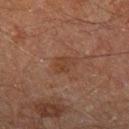Automated image analysis of the tile measured a border-irregularity index near 3.5/10 and peripheral color asymmetry of about 1.
The lesion is located on the right thigh.
A 15 mm close-up tile from a total-body photography series done for melanoma screening.
Imaged with cross-polarized lighting.
The lesion's longest dimension is about 2.5 mm.
A male subject roughly 60 years of age.